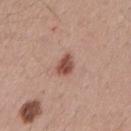<case>
  <biopsy_status>not biopsied; imaged during a skin examination</biopsy_status>
  <site>left upper arm</site>
  <image>
    <source>total-body photography crop</source>
    <field_of_view_mm>15</field_of_view_mm>
  </image>
  <patient>
    <sex>male</sex>
    <age_approx>40</age_approx>
  </patient>
</case>